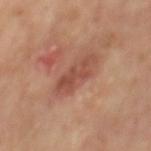Q: Was a biopsy performed?
A: catalogued during a skin exam; not biopsied
Q: What lighting was used for the tile?
A: cross-polarized illumination
Q: What kind of image is this?
A: 15 mm crop, total-body photography
Q: Patient demographics?
A: male, approximately 65 years of age
Q: Lesion location?
A: the mid back
Q: How large is the lesion?
A: ≈4.5 mm